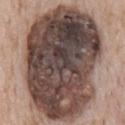follow-up = no biopsy performed (imaged during a skin exam); body site = the chest; patient = male, roughly 65 years of age; acquisition = total-body-photography crop, ~15 mm field of view.